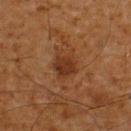workup: catalogued during a skin exam; not biopsied | imaging modality: ~15 mm crop, total-body skin-cancer survey | body site: the upper back | patient: male, aged 58–62 | illumination: cross-polarized.